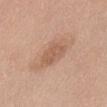notes: imaged on a skin check; not biopsied
lighting: white-light
diameter: ≈3.5 mm
site: the abdomen
subject: female, aged 58–62
image source: ~15 mm tile from a whole-body skin photo